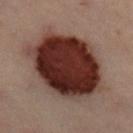Image and clinical context: Cropped from a total-body skin-imaging series; the visible field is about 15 mm. The subject is a female aged approximately 60. On the left leg. The tile uses cross-polarized illumination. An algorithmic analysis of the crop reported a border-irregularity index near 1.5/10, internal color variation of about 5.5 on a 0–10 scale, and radial color variation of about 1.5.